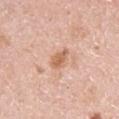Findings:
• notes · imaged on a skin check; not biopsied
• patient · female, about 50 years old
• body site · the arm
• TBP lesion metrics · an area of roughly 3.5 mm², a shape eccentricity near 0.8, and a symmetry-axis asymmetry near 0.25; border irregularity of about 3 on a 0–10 scale and a color-variation rating of about 1.5/10; a nevus-likeness score of about 75/100
• imaging modality · ~15 mm tile from a whole-body skin photo
• tile lighting · white-light illumination
• lesion size · ≈3 mm